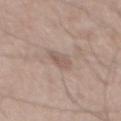workup = imaged on a skin check; not biopsied
anatomic site = the abdomen
patient = male, aged around 50
image = total-body-photography crop, ~15 mm field of view
lesion diameter = ≈2.5 mm